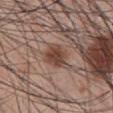{
  "biopsy_status": "not biopsied; imaged during a skin examination",
  "patient": {
    "sex": "male",
    "age_approx": 45
  },
  "site": "abdomen",
  "image": {
    "source": "total-body photography crop",
    "field_of_view_mm": 15
  }
}